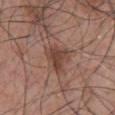site: the chest; imaging modality: total-body-photography crop, ~15 mm field of view; subject: male, in their 70s.